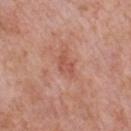workup: total-body-photography surveillance lesion; no biopsy
automated lesion analysis: roughly 7 lightness units darker than nearby skin and a normalized border contrast of about 5.5; border irregularity of about 4 on a 0–10 scale, a within-lesion color-variation index near 0.5/10, and radial color variation of about 0
size: ~2.5 mm (longest diameter)
body site: the front of the torso
patient: male, aged approximately 60
illumination: white-light illumination
image source: total-body-photography crop, ~15 mm field of view